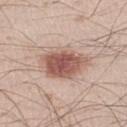Findings:
• lesion diameter · about 6 mm
• illumination · white-light illumination
• body site · the leg
• image · ~15 mm crop, total-body skin-cancer survey
• subject · male, aged 23–27